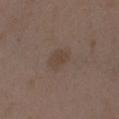The recorded lesion diameter is about 2.5 mm. A female patient, about 35 years old. This is a white-light tile. A lesion tile, about 15 mm wide, cut from a 3D total-body photograph. Automated image analysis of the tile measured a footprint of about 4 mm², an outline eccentricity of about 0.75 (0 = round, 1 = elongated), and a symmetry-axis asymmetry near 0.25. The analysis additionally found a normalized lesion–skin contrast near 5.5. And it measured a border-irregularity rating of about 2/10, a within-lesion color-variation index near 2/10, and radial color variation of about 0.5. And it measured a nevus-likeness score of about 10/100 and lesion-presence confidence of about 100/100. The lesion is on the left upper arm.